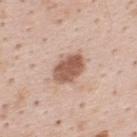Clinical impression:
No biopsy was performed on this lesion — it was imaged during a full skin examination and was not determined to be concerning.
Image and clinical context:
This image is a 15 mm lesion crop taken from a total-body photograph. On the upper back. A male subject aged 33 to 37.